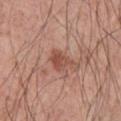<lesion>
<biopsy_status>not biopsied; imaged during a skin examination</biopsy_status>
<image>
  <source>total-body photography crop</source>
  <field_of_view_mm>15</field_of_view_mm>
</image>
<patient>
  <sex>male</sex>
  <age_approx>55</age_approx>
</patient>
<lesion_size>
  <long_diameter_mm_approx>3.0</long_diameter_mm_approx>
</lesion_size>
<site>chest</site>
<lighting>white-light</lighting>
<automated_metrics>
  <cielab_L>50</cielab_L>
  <cielab_a>24</cielab_a>
  <cielab_b>28</cielab_b>
  <vs_skin_contrast_norm>7.0</vs_skin_contrast_norm>
  <nevus_likeness_0_100>45</nevus_likeness_0_100>
  <lesion_detection_confidence_0_100>100</lesion_detection_confidence_0_100>
</automated_metrics>
</lesion>